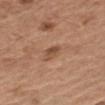Clinical impression: Part of a total-body skin-imaging series; this lesion was reviewed on a skin check and was not flagged for biopsy. Acquisition and patient details: A region of skin cropped from a whole-body photographic capture, roughly 15 mm wide. The patient is a male in their 70s. Located on the left upper arm. The total-body-photography lesion software estimated an area of roughly 3 mm², an outline eccentricity of about 0.85 (0 = round, 1 = elongated), and a symmetry-axis asymmetry near 0.4. The software also gave an average lesion color of about L≈49 a*≈21 b*≈31 (CIELAB), roughly 9 lightness units darker than nearby skin, and a normalized lesion–skin contrast near 7. The analysis additionally found a nevus-likeness score of about 30/100 and lesion-presence confidence of about 100/100. The recorded lesion diameter is about 2.5 mm. The tile uses white-light illumination.